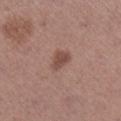Impression: The lesion was tiled from a total-body skin photograph and was not biopsied. Clinical summary: From the right lower leg. A female subject aged 63–67. A lesion tile, about 15 mm wide, cut from a 3D total-body photograph.